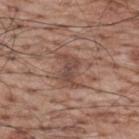| key | value |
|---|---|
| biopsy status | imaged on a skin check; not biopsied |
| site | the upper back |
| TBP lesion metrics | a lesion color around L≈48 a*≈19 b*≈25 in CIELAB, a lesion–skin lightness drop of about 8, and a normalized lesion–skin contrast near 6 |
| patient | male, roughly 70 years of age |
| acquisition | ~15 mm tile from a whole-body skin photo |